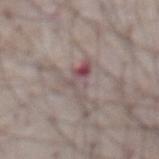The lesion was photographed on a routine skin check and not biopsied; there is no pathology result. The tile uses white-light illumination. A close-up tile cropped from a whole-body skin photograph, about 15 mm across. A male patient, in their mid-70s. Automated tile analysis of the lesion measured a footprint of about 6 mm², an eccentricity of roughly 0.85, and two-axis asymmetry of about 0.55. Located on the abdomen. The recorded lesion diameter is about 5 mm.